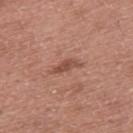biopsy status=imaged on a skin check; not biopsied
imaging modality=~15 mm tile from a whole-body skin photo
subject=male, roughly 60 years of age
illumination=white-light illumination
image-analysis metrics=an average lesion color of about L≈49 a*≈24 b*≈27 (CIELAB), roughly 10 lightness units darker than nearby skin, and a normalized lesion–skin contrast near 7; a border-irregularity rating of about 4/10, internal color variation of about 1.5 on a 0–10 scale, and a peripheral color-asymmetry measure near 0; an automated nevus-likeness rating near 10 out of 100 and a detector confidence of about 100 out of 100 that the crop contains a lesion
anatomic site=the upper back